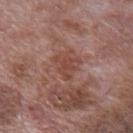Clinical impression: Captured during whole-body skin photography for melanoma surveillance; the lesion was not biopsied. Context: Imaged with white-light lighting. The patient is a male aged approximately 70. A 15 mm close-up tile from a total-body photography series done for melanoma screening. The lesion is on the back.